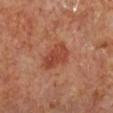Imaged during a routine full-body skin examination; the lesion was not biopsied and no histopathology is available.
From the right lower leg.
About 4 mm across.
A male subject, about 65 years old.
Automated image analysis of the tile measured a lesion area of about 8 mm², an outline eccentricity of about 0.8 (0 = round, 1 = elongated), and two-axis asymmetry of about 0.25. The software also gave an average lesion color of about L≈45 a*≈29 b*≈33 (CIELAB) and a lesion–skin lightness drop of about 9. The software also gave a border-irregularity rating of about 2.5/10, internal color variation of about 3.5 on a 0–10 scale, and peripheral color asymmetry of about 1. It also reported a classifier nevus-likeness of about 85/100 and a detector confidence of about 100 out of 100 that the crop contains a lesion.
Cropped from a total-body skin-imaging series; the visible field is about 15 mm.
The tile uses cross-polarized illumination.